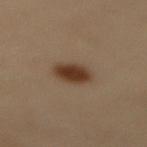Recorded during total-body skin imaging; not selected for excision or biopsy. The patient is a female aged 48 to 52. The lesion is located on the mid back. The tile uses cross-polarized illumination. Automated image analysis of the tile measured a lesion color around L≈32 a*≈14 b*≈24 in CIELAB, a lesion–skin lightness drop of about 11, and a normalized lesion–skin contrast near 10.5. And it measured a detector confidence of about 100 out of 100 that the crop contains a lesion. A roughly 15 mm field-of-view crop from a total-body skin photograph. Longest diameter approximately 3.5 mm.